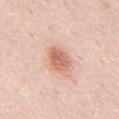This lesion was catalogued during total-body skin photography and was not selected for biopsy. Automated tile analysis of the lesion measured an area of roughly 7.5 mm², an outline eccentricity of about 0.6 (0 = round, 1 = elongated), and two-axis asymmetry of about 0.2. The software also gave a within-lesion color-variation index near 3.5/10. The software also gave an automated nevus-likeness rating near 100 out of 100. A roughly 15 mm field-of-view crop from a total-body skin photograph. The subject is a male roughly 50 years of age. The recorded lesion diameter is about 3.5 mm. The lesion is on the mid back.